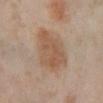No biopsy was performed on this lesion — it was imaged during a full skin examination and was not determined to be concerning.
A female subject, in their mid- to late 50s.
From the left lower leg.
A 15 mm close-up extracted from a 3D total-body photography capture.
About 5 mm across.
This is a cross-polarized tile.
Automated image analysis of the tile measured a footprint of about 17 mm², an outline eccentricity of about 0.45 (0 = round, 1 = elongated), and a symmetry-axis asymmetry near 0.3. The analysis additionally found border irregularity of about 3 on a 0–10 scale and a within-lesion color-variation index near 3.5/10.